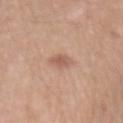Case summary:
• workup — imaged on a skin check; not biopsied
• subject — male, aged 63 to 67
• site — the lower back
• image source — ~15 mm tile from a whole-body skin photo
• illumination — white-light
• size — about 2.5 mm